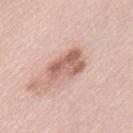<tbp_lesion>
  <biopsy_status>not biopsied; imaged during a skin examination</biopsy_status>
  <site>left thigh</site>
  <patient>
    <sex>female</sex>
    <age_approx>65</age_approx>
  </patient>
  <image>
    <source>total-body photography crop</source>
    <field_of_view_mm>15</field_of_view_mm>
  </image>
</tbp_lesion>